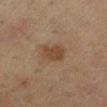This lesion was catalogued during total-body skin photography and was not selected for biopsy. The lesion is on the left lower leg. Automated tile analysis of the lesion measured a mean CIELAB color near L≈39 a*≈14 b*≈28 and a normalized border contrast of about 7. It also reported an automated nevus-likeness rating near 35 out of 100. This image is a 15 mm lesion crop taken from a total-body photograph. A female subject aged 53 to 57. Imaged with cross-polarized lighting.